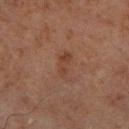| key | value |
|---|---|
| biopsy status | total-body-photography surveillance lesion; no biopsy |
| lesion size | ~3 mm (longest diameter) |
| patient | male, approximately 65 years of age |
| automated lesion analysis | a color-variation rating of about 2.5/10 and peripheral color asymmetry of about 0.5; an automated nevus-likeness rating near 0 out of 100 and a lesion-detection confidence of about 100/100 |
| image source | total-body-photography crop, ~15 mm field of view |
| tile lighting | cross-polarized illumination |
| site | the left lower leg |